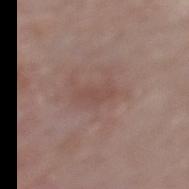Findings:
– biopsy status — imaged on a skin check; not biopsied
– automated lesion analysis — an average lesion color of about L≈48 a*≈19 b*≈23 (CIELAB), a lesion–skin lightness drop of about 6, and a lesion-to-skin contrast of about 5 (normalized; higher = more distinct); border irregularity of about 3.5 on a 0–10 scale and a within-lesion color-variation index near 0.5/10
– location — the mid back
– illumination — white-light illumination
– imaging modality — total-body-photography crop, ~15 mm field of view
– lesion size — about 3.5 mm
– subject — male, aged 78–82